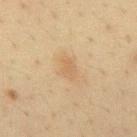Impression: The lesion was tiled from a total-body skin photograph and was not biopsied.